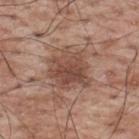notes: catalogued during a skin exam; not biopsied | image-analysis metrics: an area of roughly 16 mm², an outline eccentricity of about 0.4 (0 = round, 1 = elongated), and a shape-asymmetry score of about 0.25 (0 = symmetric); a within-lesion color-variation index near 4.5/10 | patient: male, in their 70s | image source: ~15 mm crop, total-body skin-cancer survey | location: the upper back | lighting: white-light illumination.